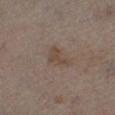{"biopsy_status": "not biopsied; imaged during a skin examination", "automated_metrics": {"cielab_L": 43, "cielab_a": 13, "cielab_b": 23, "border_irregularity_0_10": 5.5, "color_variation_0_10": 1.5, "peripheral_color_asymmetry": 0.5}, "patient": {"sex": "male", "age_approx": 50}, "image": {"source": "total-body photography crop", "field_of_view_mm": 15}, "lighting": "cross-polarized", "site": "left leg"}